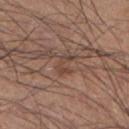- notes: imaged on a skin check; not biopsied
- subject: male, aged 33–37
- size: ≈3 mm
- TBP lesion metrics: an outline eccentricity of about 0.75 (0 = round, 1 = elongated) and two-axis asymmetry of about 0.5; an automated nevus-likeness rating near 0 out of 100 and a lesion-detection confidence of about 55/100
- imaging modality: total-body-photography crop, ~15 mm field of view
- tile lighting: white-light
- location: the left lower leg